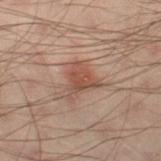Assessment: Recorded during total-body skin imaging; not selected for excision or biopsy. Image and clinical context: Located on the left thigh. A close-up tile cropped from a whole-body skin photograph, about 15 mm across. A male patient, in their 50s.